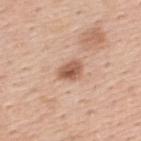Captured during whole-body skin photography for melanoma surveillance; the lesion was not biopsied.
A 15 mm close-up tile from a total-body photography series done for melanoma screening.
The tile uses white-light illumination.
A male subject aged 53–57.
Longest diameter approximately 3 mm.
Located on the upper back.